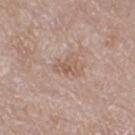The lesion was photographed on a routine skin check and not biopsied; there is no pathology result. From the right thigh. The tile uses white-light illumination. Automated tile analysis of the lesion measured a footprint of about 3.5 mm² and an eccentricity of roughly 0.8. The analysis additionally found a lesion–skin lightness drop of about 7 and a normalized border contrast of about 5.5. A 15 mm close-up tile from a total-body photography series done for melanoma screening. A female subject, roughly 40 years of age. The lesion's longest dimension is about 2.5 mm.